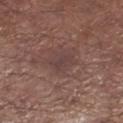Case summary:
* notes — imaged on a skin check; not biopsied
* imaging modality — 15 mm crop, total-body photography
* anatomic site — the leg
* patient — male, aged 53 to 57